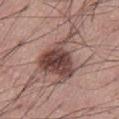Recorded during total-body skin imaging; not selected for excision or biopsy.
The lesion is on the abdomen.
The subject is a male in their mid-50s.
A 15 mm close-up tile from a total-body photography series done for melanoma screening.
Longest diameter approximately 7.5 mm.